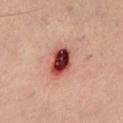Assessment:
Part of a total-body skin-imaging series; this lesion was reviewed on a skin check and was not flagged for biopsy.
Context:
The lesion is located on the left lower leg. Longest diameter approximately 4 mm. This is a white-light tile. The patient is a male aged approximately 35. A 15 mm close-up tile from a total-body photography series done for melanoma screening.